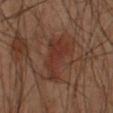Impression:
Captured during whole-body skin photography for melanoma surveillance; the lesion was not biopsied.
Image and clinical context:
A 15 mm crop from a total-body photograph taken for skin-cancer surveillance. Approximately 5 mm at its widest. The subject is a male aged approximately 50. The lesion is located on the left arm.